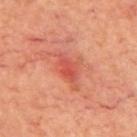From the upper back. A male subject, approximately 70 years of age. A lesion tile, about 15 mm wide, cut from a 3D total-body photograph.